A 15 mm close-up extracted from a 3D total-body photography capture. Located on the leg. A male patient aged around 75: 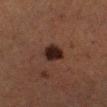The biopsy diagnosis was a melanoma in situ.